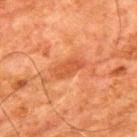biopsy status = catalogued during a skin exam; not biopsied
image = 15 mm crop, total-body photography
lesion diameter = about 3.5 mm
illumination = cross-polarized illumination
subject = male, roughly 80 years of age
anatomic site = the upper back